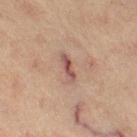The lesion was tiled from a total-body skin photograph and was not biopsied.
Cropped from a total-body skin-imaging series; the visible field is about 15 mm.
A female subject, in their mid- to late 60s.
The lesion is located on the right thigh.
Captured under cross-polarized illumination.
The lesion's longest dimension is about 3.5 mm.